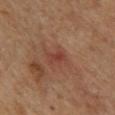notes — no biopsy performed (imaged during a skin exam); acquisition — ~15 mm crop, total-body skin-cancer survey; subject — male, approximately 85 years of age or older; location — the back; lesion size — ~2.5 mm (longest diameter); illumination — cross-polarized illumination.